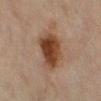Impression: Captured during whole-body skin photography for melanoma surveillance; the lesion was not biopsied. Acquisition and patient details: The lesion is located on the leg. A lesion tile, about 15 mm wide, cut from a 3D total-body photograph. The patient is a female aged around 70. Automated tile analysis of the lesion measured a mean CIELAB color near L≈37 a*≈18 b*≈28, about 12 CIELAB-L* units darker than the surrounding skin, and a normalized lesion–skin contrast near 11. It also reported radial color variation of about 1.5. The software also gave a classifier nevus-likeness of about 100/100 and lesion-presence confidence of about 100/100.